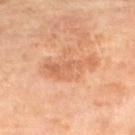{
  "biopsy_status": "not biopsied; imaged during a skin examination",
  "image": {
    "source": "total-body photography crop",
    "field_of_view_mm": 15
  },
  "patient": {
    "sex": "female",
    "age_approx": 70
  },
  "site": "left lower leg"
}